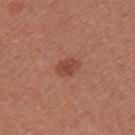Part of a total-body skin-imaging series; this lesion was reviewed on a skin check and was not flagged for biopsy. A roughly 15 mm field-of-view crop from a total-body skin photograph. A female patient roughly 25 years of age. The tile uses white-light illumination. On the arm.